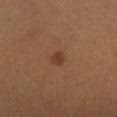{"biopsy_status": "not biopsied; imaged during a skin examination", "site": "left lower leg", "patient": {"sex": "female", "age_approx": 40}, "image": {"source": "total-body photography crop", "field_of_view_mm": 15}}